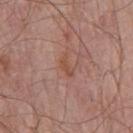The lesion was tiled from a total-body skin photograph and was not biopsied.
Imaged with white-light lighting.
The lesion's longest dimension is about 3 mm.
A male subject aged around 75.
This image is a 15 mm lesion crop taken from a total-body photograph.
The total-body-photography lesion software estimated an area of roughly 3 mm², an outline eccentricity of about 0.9 (0 = round, 1 = elongated), and two-axis asymmetry of about 0.5. It also reported border irregularity of about 5 on a 0–10 scale, a color-variation rating of about 1/10, and radial color variation of about 0.
From the chest.